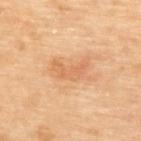This lesion was catalogued during total-body skin photography and was not selected for biopsy.
Captured under cross-polarized illumination.
A 15 mm close-up tile from a total-body photography series done for melanoma screening.
An algorithmic analysis of the crop reported a lesion area of about 6.5 mm², a shape eccentricity near 0.9, and a symmetry-axis asymmetry near 0.5. And it measured an average lesion color of about L≈65 a*≈24 b*≈40 (CIELAB), roughly 8 lightness units darker than nearby skin, and a lesion-to-skin contrast of about 5.5 (normalized; higher = more distinct). And it measured border irregularity of about 5.5 on a 0–10 scale, a color-variation rating of about 2/10, and radial color variation of about 0.5.
The lesion is located on the upper back.
A female subject, aged approximately 65.
The recorded lesion diameter is about 4 mm.